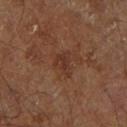workup = no biopsy performed (imaged during a skin exam); patient = male, aged approximately 60; image = ~15 mm crop, total-body skin-cancer survey; location = the right leg; lesion diameter = ~3 mm (longest diameter).